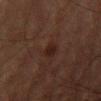patient: male, in their mid- to late 80s or older
automated metrics: a shape eccentricity near 0.85; a lesion–skin lightness drop of about 6 and a normalized lesion–skin contrast near 8; a within-lesion color-variation index near 0.5/10 and a peripheral color-asymmetry measure near 0; a classifier nevus-likeness of about 10/100 and lesion-presence confidence of about 100/100
site: the right thigh
image: 15 mm crop, total-body photography
size: ≈3 mm